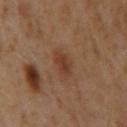This lesion was catalogued during total-body skin photography and was not selected for biopsy. The subject is a male about 60 years old. The lesion is on the mid back. A lesion tile, about 15 mm wide, cut from a 3D total-body photograph.